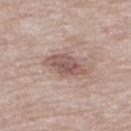biopsy status: no biopsy performed (imaged during a skin exam); subject: male, in their mid- to late 70s; anatomic site: the mid back; acquisition: ~15 mm tile from a whole-body skin photo.